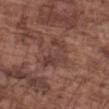Case summary:
• biopsy status · no biopsy performed (imaged during a skin exam)
• tile lighting · white-light illumination
• size · about 5 mm
• imaging modality · 15 mm crop, total-body photography
• site · the left forearm
• subject · male, aged 73 to 77
• automated lesion analysis · an area of roughly 11 mm² and a shape-asymmetry score of about 0.45 (0 = symmetric); an average lesion color of about L≈40 a*≈19 b*≈23 (CIELAB) and a normalized border contrast of about 6; a border-irregularity index near 5.5/10, a within-lesion color-variation index near 4/10, and a peripheral color-asymmetry measure near 1.5